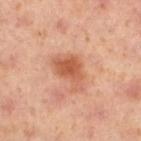Imaged during a routine full-body skin examination; the lesion was not biopsied and no histopathology is available.